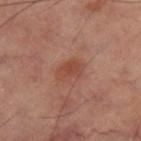The lesion is on the right thigh. A lesion tile, about 15 mm wide, cut from a 3D total-body photograph. Captured under cross-polarized illumination. The total-body-photography lesion software estimated a mean CIELAB color near L≈47 a*≈25 b*≈30, a lesion–skin lightness drop of about 8, and a normalized lesion–skin contrast near 6. The software also gave a border-irregularity index near 2.5/10, a within-lesion color-variation index near 3/10, and peripheral color asymmetry of about 1. About 3 mm across.